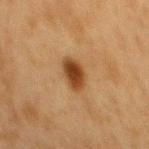Findings:
* biopsy status: imaged on a skin check; not biopsied
* illumination: cross-polarized illumination
* imaging modality: ~15 mm crop, total-body skin-cancer survey
* anatomic site: the mid back
* lesion size: about 4 mm
* subject: male, about 75 years old